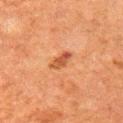{
  "biopsy_status": "not biopsied; imaged during a skin examination",
  "patient": {
    "sex": "male",
    "age_approx": 60
  },
  "image": {
    "source": "total-body photography crop",
    "field_of_view_mm": 15
  },
  "site": "right upper arm",
  "lighting": "cross-polarized",
  "automated_metrics": {
    "area_mm2_approx": 4.0,
    "eccentricity": 0.85,
    "shape_asymmetry": 0.25,
    "cielab_L": 42,
    "cielab_a": 24,
    "cielab_b": 32,
    "vs_skin_darker_L": 9.0,
    "vs_skin_contrast_norm": 7.5,
    "border_irregularity_0_10": 3.0,
    "peripheral_color_asymmetry": 1.5
  },
  "lesion_size": {
    "long_diameter_mm_approx": 3.0
  }
}